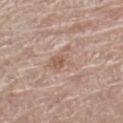follow-up: catalogued during a skin exam; not biopsied | patient: female, about 65 years old | image-analysis metrics: an area of roughly 8.5 mm², an outline eccentricity of about 0.75 (0 = round, 1 = elongated), and two-axis asymmetry of about 0.4; a border-irregularity index near 4.5/10, a within-lesion color-variation index near 4.5/10, and a peripheral color-asymmetry measure near 1.5 | illumination: white-light | anatomic site: the left thigh | image source: 15 mm crop, total-body photography.